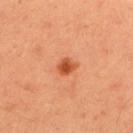Clinical impression:
No biopsy was performed on this lesion — it was imaged during a full skin examination and was not determined to be concerning.
Clinical summary:
The total-body-photography lesion software estimated an area of roughly 4 mm² and a shape eccentricity near 0.6. The analysis additionally found an average lesion color of about L≈52 a*≈32 b*≈41 (CIELAB), about 12 CIELAB-L* units darker than the surrounding skin, and a normalized lesion–skin contrast near 9. The software also gave a color-variation rating of about 3/10 and a peripheral color-asymmetry measure near 1. The tile uses cross-polarized illumination. On the upper back. This image is a 15 mm lesion crop taken from a total-body photograph. The recorded lesion diameter is about 2.5 mm. The patient is a male in their mid- to late 30s.